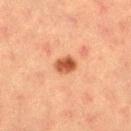Assessment:
The lesion was tiled from a total-body skin photograph and was not biopsied.
Background:
About 2.5 mm across. Automated tile analysis of the lesion measured an area of roughly 4.5 mm² and an outline eccentricity of about 0.65 (0 = round, 1 = elongated). The lesion is on the left thigh. The patient is a female aged approximately 55. This image is a 15 mm lesion crop taken from a total-body photograph.